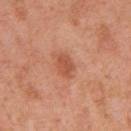{
  "biopsy_status": "not biopsied; imaged during a skin examination",
  "lesion_size": {
    "long_diameter_mm_approx": 3.0
  },
  "patient": {
    "sex": "female",
    "age_approx": 40
  },
  "image": {
    "source": "total-body photography crop",
    "field_of_view_mm": 15
  },
  "automated_metrics": {
    "eccentricity": 0.7,
    "shape_asymmetry": 0.15,
    "cielab_L": 55,
    "cielab_a": 28,
    "cielab_b": 35,
    "vs_skin_darker_L": 9.0,
    "vs_skin_contrast_norm": 6.5,
    "nevus_likeness_0_100": 55
  },
  "site": "right upper arm",
  "lighting": "white-light"
}